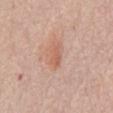anatomic site = the chest | size = ~2.5 mm (longest diameter) | automated lesion analysis = a footprint of about 3 mm² and an eccentricity of roughly 0.9; a lesion color around L≈60 a*≈24 b*≈31 in CIELAB, about 8 CIELAB-L* units darker than the surrounding skin, and a normalized lesion–skin contrast near 6 | patient = female, aged around 65 | acquisition = ~15 mm tile from a whole-body skin photo | illumination = white-light.